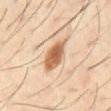Findings:
– follow-up · imaged on a skin check; not biopsied
– image · 15 mm crop, total-body photography
– tile lighting · cross-polarized illumination
– lesion size · ~4.5 mm (longest diameter)
– site · the abdomen
– image-analysis metrics · an area of roughly 9.5 mm² and an outline eccentricity of about 0.85 (0 = round, 1 = elongated); a nevus-likeness score of about 100/100 and lesion-presence confidence of about 100/100
– subject · male, approximately 40 years of age